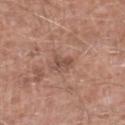tile lighting: white-light | image: 15 mm crop, total-body photography | patient: male, aged 58 to 62 | automated lesion analysis: an area of roughly 3 mm² and a shape eccentricity near 0.8; a normalized border contrast of about 6.5; a nevus-likeness score of about 0/100 and lesion-presence confidence of about 100/100 | anatomic site: the left lower leg | lesion size: about 2.5 mm.